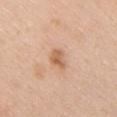This lesion was catalogued during total-body skin photography and was not selected for biopsy.
The lesion is located on the chest.
This image is a 15 mm lesion crop taken from a total-body photograph.
This is a white-light tile.
A male patient aged 58–62.
Longest diameter approximately 2.5 mm.
The lesion-visualizer software estimated a footprint of about 4 mm², a shape eccentricity near 0.65, and a shape-asymmetry score of about 0.35 (0 = symmetric). The software also gave about 10 CIELAB-L* units darker than the surrounding skin and a normalized border contrast of about 7.5. The software also gave a border-irregularity index near 3.5/10, a color-variation rating of about 3.5/10, and radial color variation of about 1.5. And it measured lesion-presence confidence of about 100/100.